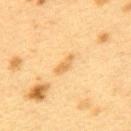The lesion was tiled from a total-body skin photograph and was not biopsied.
A 15 mm close-up tile from a total-body photography series done for melanoma screening.
Automated tile analysis of the lesion measured an area of roughly 3 mm² and a symmetry-axis asymmetry near 0.35. And it measured a border-irregularity rating of about 4/10, a color-variation rating of about 0/10, and a peripheral color-asymmetry measure near 0. The software also gave a nevus-likeness score of about 15/100 and a lesion-detection confidence of about 100/100.
From the upper back.
The recorded lesion diameter is about 3 mm.
The subject is a female about 40 years old.
Imaged with cross-polarized lighting.